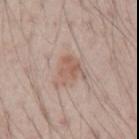Recorded during total-body skin imaging; not selected for excision or biopsy. This is a white-light tile. The lesion's longest dimension is about 3.5 mm. A roughly 15 mm field-of-view crop from a total-body skin photograph. The lesion is located on the arm. A male patient aged around 70.